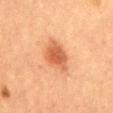Assessment: This lesion was catalogued during total-body skin photography and was not selected for biopsy. Acquisition and patient details: Automated tile analysis of the lesion measured an automated nevus-likeness rating near 95 out of 100 and a detector confidence of about 100 out of 100 that the crop contains a lesion. Imaged with cross-polarized lighting. A female subject, about 60 years old. A lesion tile, about 15 mm wide, cut from a 3D total-body photograph. Measured at roughly 4.5 mm in maximum diameter. From the abdomen.